The tile uses white-light illumination. From the chest. A lesion tile, about 15 mm wide, cut from a 3D total-body photograph. A female subject in their mid- to late 70s — 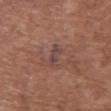Conclusion: Biopsy histopathology demonstrated a nodular basal cell carcinoma, classified as a skin cancer.